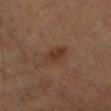{
  "biopsy_status": "not biopsied; imaged during a skin examination",
  "patient": {
    "sex": "male",
    "age_approx": 55
  },
  "image": {
    "source": "total-body photography crop",
    "field_of_view_mm": 15
  },
  "lighting": "cross-polarized",
  "site": "leg"
}